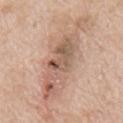This lesion was catalogued during total-body skin photography and was not selected for biopsy. Measured at roughly 12 mm in maximum diameter. A male patient aged 63–67. A lesion tile, about 15 mm wide, cut from a 3D total-body photograph. On the chest. Imaged with white-light lighting.